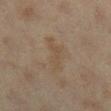Assessment: No biopsy was performed on this lesion — it was imaged during a full skin examination and was not determined to be concerning. Context: This image is a 15 mm lesion crop taken from a total-body photograph. A female subject in their 40s. Measured at roughly 4.5 mm in maximum diameter. From the right lower leg. This is a cross-polarized tile. Automated tile analysis of the lesion measured a footprint of about 7.5 mm² and two-axis asymmetry of about 0.55.